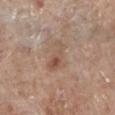This lesion was catalogued during total-body skin photography and was not selected for biopsy.